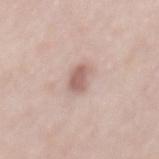workup: no biopsy performed (imaged during a skin exam) | lesion size: ≈3.5 mm | acquisition: ~15 mm crop, total-body skin-cancer survey | body site: the mid back | patient: female, aged 28 to 32 | lighting: white-light illumination.